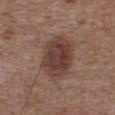biopsy status=no biopsy performed (imaged during a skin exam) | image=total-body-photography crop, ~15 mm field of view | lighting=white-light | lesion diameter=~5.5 mm (longest diameter) | automated metrics=a border-irregularity rating of about 1.5/10, a color-variation rating of about 4/10, and a peripheral color-asymmetry measure near 1 | location=the upper back | patient=male, approximately 50 years of age.